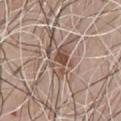The lesion was tiled from a total-body skin photograph and was not biopsied. A region of skin cropped from a whole-body photographic capture, roughly 15 mm wide. From the front of the torso. This is a white-light tile. The patient is a male aged 63 to 67. Measured at roughly 4 mm in maximum diameter.